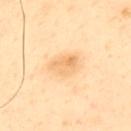Part of a total-body skin-imaging series; this lesion was reviewed on a skin check and was not flagged for biopsy. A 15 mm crop from a total-body photograph taken for skin-cancer surveillance. The lesion is on the upper back. Automated image analysis of the tile measured a lesion–skin lightness drop of about 9. It also reported a border-irregularity index near 2.5/10 and a peripheral color-asymmetry measure near 2. It also reported a nevus-likeness score of about 25/100 and a lesion-detection confidence of about 100/100. Imaged with cross-polarized lighting. The subject is a male roughly 50 years of age. Measured at roughly 3.5 mm in maximum diameter.